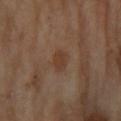<case>
  <biopsy_status>not biopsied; imaged during a skin examination</biopsy_status>
  <lighting>cross-polarized</lighting>
  <image>
    <source>total-body photography crop</source>
    <field_of_view_mm>15</field_of_view_mm>
  </image>
  <patient>
    <sex>female</sex>
    <age_approx>70</age_approx>
  </patient>
  <site>left forearm</site>
</case>